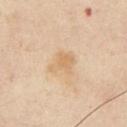follow-up: catalogued during a skin exam; not biopsied.